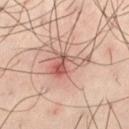Clinical impression: The lesion was tiled from a total-body skin photograph and was not biopsied. Background: Longest diameter approximately 5 mm. Automated image analysis of the tile measured a footprint of about 10 mm² and a symmetry-axis asymmetry near 0.4. It also reported a lesion color around L≈58 a*≈23 b*≈26 in CIELAB and a normalized border contrast of about 7.5. Cropped from a whole-body photographic skin survey; the tile spans about 15 mm. Located on the left thigh. The patient is a male aged around 40. Captured under cross-polarized illumination.